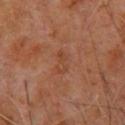Assessment:
Part of a total-body skin-imaging series; this lesion was reviewed on a skin check and was not flagged for biopsy.
Context:
About 2.5 mm across. This is a cross-polarized tile. The patient is a male aged 58 to 62. This image is a 15 mm lesion crop taken from a total-body photograph. The lesion is located on the upper back.